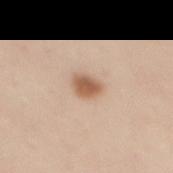Captured during whole-body skin photography for melanoma surveillance; the lesion was not biopsied. The lesion is located on the mid back. The patient is a female in their 40s. This is a white-light tile. The lesion's longest dimension is about 2.5 mm. The total-body-photography lesion software estimated a lesion area of about 5 mm², an eccentricity of roughly 0.6, and two-axis asymmetry of about 0.2. And it measured a lesion color around L≈59 a*≈20 b*≈32 in CIELAB, a lesion–skin lightness drop of about 14, and a lesion-to-skin contrast of about 9 (normalized; higher = more distinct). The analysis additionally found an automated nevus-likeness rating near 100 out of 100 and lesion-presence confidence of about 100/100. A 15 mm close-up extracted from a 3D total-body photography capture.